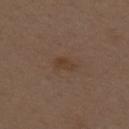{"biopsy_status": "not biopsied; imaged during a skin examination"}